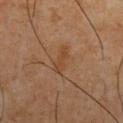workup: imaged on a skin check; not biopsied
imaging modality: total-body-photography crop, ~15 mm field of view
lesion size: ~3.5 mm (longest diameter)
subject: male, in their mid- to late 60s
body site: the front of the torso